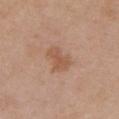| key | value |
|---|---|
| workup | total-body-photography surveillance lesion; no biopsy |
| acquisition | ~15 mm crop, total-body skin-cancer survey |
| site | the chest |
| size | ≈3.5 mm |
| illumination | white-light illumination |
| patient | female, aged approximately 40 |